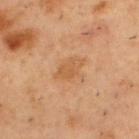Assessment: Part of a total-body skin-imaging series; this lesion was reviewed on a skin check and was not flagged for biopsy. Clinical summary: The lesion is located on the upper back. Imaged with cross-polarized lighting. A male subject, aged approximately 55. This image is a 15 mm lesion crop taken from a total-body photograph. Approximately 3.5 mm at its widest.